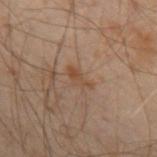follow-up — no biopsy performed (imaged during a skin exam) | image source — 15 mm crop, total-body photography | body site — the left forearm | size — ≈3 mm | patient — male, aged 48 to 52 | automated lesion analysis — an area of roughly 3.5 mm² and a shape eccentricity near 0.9; a lesion color around L≈37 a*≈15 b*≈25 in CIELAB and about 6 CIELAB-L* units darker than the surrounding skin; a peripheral color-asymmetry measure near 0.5; an automated nevus-likeness rating near 0 out of 100 and a detector confidence of about 100 out of 100 that the crop contains a lesion.